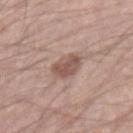Impression: The lesion was tiled from a total-body skin photograph and was not biopsied. Image and clinical context: A male subject about 45 years old. This is a white-light tile. Longest diameter approximately 3 mm. The lesion is on the arm. A 15 mm close-up tile from a total-body photography series done for melanoma screening.